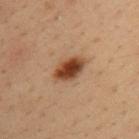The lesion was photographed on a routine skin check and not biopsied; there is no pathology result. On the upper back. A 15 mm close-up extracted from a 3D total-body photography capture. This is a cross-polarized tile. The total-body-photography lesion software estimated a lesion area of about 7.5 mm² and a shape-asymmetry score of about 0.15 (0 = symmetric). It also reported a mean CIELAB color near L≈40 a*≈21 b*≈31, about 15 CIELAB-L* units darker than the surrounding skin, and a normalized border contrast of about 12. It also reported a border-irregularity index near 1.5/10, internal color variation of about 5 on a 0–10 scale, and a peripheral color-asymmetry measure near 1.5. The patient is a male aged approximately 35.